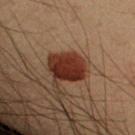site: the right forearm
patient: male, in their mid-50s
imaging modality: 15 mm crop, total-body photography